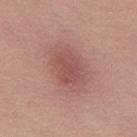  biopsy_status: not biopsied; imaged during a skin examination
  patient:
    sex: female
    age_approx: 35
  site: left thigh
  lesion_size:
    long_diameter_mm_approx: 4.0
  image:
    source: total-body photography crop
    field_of_view_mm: 15
  lighting: white-light